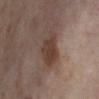biopsy status — total-body-photography surveillance lesion; no biopsy
patient — female, aged 53–57
lighting — cross-polarized illumination
lesion diameter — ≈4 mm
image — 15 mm crop, total-body photography
body site — the left lower leg
automated lesion analysis — an area of roughly 10 mm², a shape eccentricity near 0.65, and two-axis asymmetry of about 0.3; roughly 9 lightness units darker than nearby skin and a normalized lesion–skin contrast near 8; a within-lesion color-variation index near 3/10 and peripheral color asymmetry of about 1; a classifier nevus-likeness of about 15/100 and a lesion-detection confidence of about 100/100